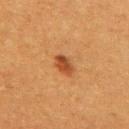Clinical impression: Part of a total-body skin-imaging series; this lesion was reviewed on a skin check and was not flagged for biopsy. Context: A 15 mm close-up extracted from a 3D total-body photography capture. Longest diameter approximately 3 mm. From the arm. Imaged with cross-polarized lighting. Automated tile analysis of the lesion measured a lesion area of about 4 mm², an outline eccentricity of about 0.8 (0 = round, 1 = elongated), and two-axis asymmetry of about 0.25. The analysis additionally found about 10 CIELAB-L* units darker than the surrounding skin. And it measured an automated nevus-likeness rating near 95 out of 100 and lesion-presence confidence of about 100/100. The subject is a female aged around 40.